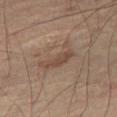{"biopsy_status": "not biopsied; imaged during a skin examination"}